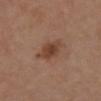The lesion is located on the chest.
A roughly 15 mm field-of-view crop from a total-body skin photograph.
A female patient, in their 40s.